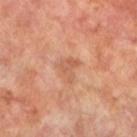Notes:
– follow-up: catalogued during a skin exam; not biopsied
– tile lighting: cross-polarized illumination
– TBP lesion metrics: an outline eccentricity of about 0.55 (0 = round, 1 = elongated) and a shape-asymmetry score of about 0.5 (0 = symmetric); a lesion–skin lightness drop of about 7; a detector confidence of about 100 out of 100 that the crop contains a lesion
– acquisition: total-body-photography crop, ~15 mm field of view
– anatomic site: the left lower leg
– subject: male, in their 70s